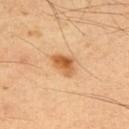Clinical impression:
Captured during whole-body skin photography for melanoma surveillance; the lesion was not biopsied.
Acquisition and patient details:
This is a cross-polarized tile. A male subject, aged 48 to 52. A 15 mm close-up extracted from a 3D total-body photography capture. From the upper back. The lesion's longest dimension is about 3.5 mm.